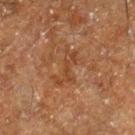{"biopsy_status": "not biopsied; imaged during a skin examination", "patient": {"sex": "male", "age_approx": 60}, "lighting": "cross-polarized", "image": {"source": "total-body photography crop", "field_of_view_mm": 15}, "site": "right lower leg"}